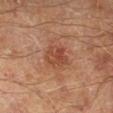• follow-up: no biopsy performed (imaged during a skin exam)
• subject: male, aged 63 to 67
• imaging modality: ~15 mm tile from a whole-body skin photo
• body site: the right lower leg